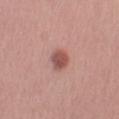Impression:
Captured during whole-body skin photography for melanoma surveillance; the lesion was not biopsied.